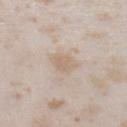– biopsy status · no biopsy performed (imaged during a skin exam)
– patient · female, in their mid-20s
– acquisition · 15 mm crop, total-body photography
– diameter · ~3.5 mm (longest diameter)
– tile lighting · white-light
– site · the left lower leg